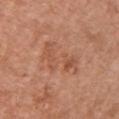Acquisition and patient details:
A female subject, aged around 65. A lesion tile, about 15 mm wide, cut from a 3D total-body photograph. The lesion is on the chest. The lesion's longest dimension is about 5.5 mm. The tile uses white-light illumination.